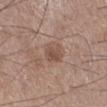Notes:
- notes: catalogued during a skin exam; not biopsied
- imaging modality: 15 mm crop, total-body photography
- diameter: about 2.5 mm
- subject: male, aged approximately 50
- illumination: white-light
- automated metrics: a lesion area of about 4.5 mm² and a shape-asymmetry score of about 0.25 (0 = symmetric); a border-irregularity index near 2.5/10, a within-lesion color-variation index near 2/10, and a peripheral color-asymmetry measure near 1
- location: the leg